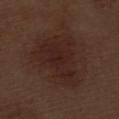Impression: The lesion was tiled from a total-body skin photograph and was not biopsied. Clinical summary: Approximately 7 mm at its widest. The patient is a male about 70 years old. A close-up tile cropped from a whole-body skin photograph, about 15 mm across. The lesion is located on the left thigh.